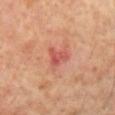Image and clinical context:
From the mid back. A male subject aged 58–62. Captured under cross-polarized illumination. A 15 mm crop from a total-body photograph taken for skin-cancer surveillance. The lesion's longest dimension is about 3 mm.